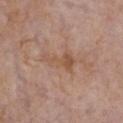Q: Is there a histopathology result?
A: imaged on a skin check; not biopsied
Q: Lesion location?
A: the front of the torso
Q: Illumination type?
A: white-light illumination
Q: Patient demographics?
A: female, aged around 85 or older
Q: What kind of image is this?
A: total-body-photography crop, ~15 mm field of view